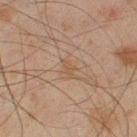Impression: Captured during whole-body skin photography for melanoma surveillance; the lesion was not biopsied. Acquisition and patient details: A 15 mm crop from a total-body photograph taken for skin-cancer surveillance. A male subject approximately 45 years of age. The lesion is on the leg. The recorded lesion diameter is about 2.5 mm. The tile uses cross-polarized illumination.